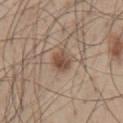Clinical impression:
This lesion was catalogued during total-body skin photography and was not selected for biopsy.
Clinical summary:
A male subject approximately 45 years of age. Imaged with white-light lighting. A region of skin cropped from a whole-body photographic capture, roughly 15 mm wide. Longest diameter approximately 2.5 mm. The lesion is on the chest.